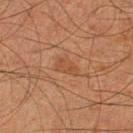This lesion was catalogued during total-body skin photography and was not selected for biopsy. The patient is a male aged around 65. Approximately 3 mm at its widest. A 15 mm crop from a total-body photograph taken for skin-cancer surveillance. The lesion is located on the leg. Imaged with cross-polarized lighting.